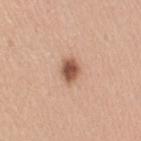Q: Was a biopsy performed?
A: total-body-photography surveillance lesion; no biopsy
Q: Lesion location?
A: the right upper arm
Q: Automated lesion metrics?
A: border irregularity of about 2.5 on a 0–10 scale, a within-lesion color-variation index near 3.5/10, and peripheral color asymmetry of about 1
Q: How large is the lesion?
A: ~3 mm (longest diameter)
Q: What lighting was used for the tile?
A: white-light illumination
Q: Who is the patient?
A: female, in their 30s
Q: What is the imaging modality?
A: ~15 mm crop, total-body skin-cancer survey